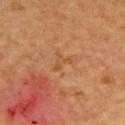The lesion was photographed on a routine skin check and not biopsied; there is no pathology result.
The lesion is located on the upper back.
A close-up tile cropped from a whole-body skin photograph, about 15 mm across.
The patient is female.